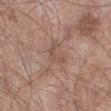Clinical impression:
The lesion was photographed on a routine skin check and not biopsied; there is no pathology result.
Context:
A 15 mm close-up extracted from a 3D total-body photography capture. The lesion is located on the left lower leg. Longest diameter approximately 3 mm. A male subject, about 55 years old. Imaged with white-light lighting.